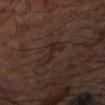The lesion was photographed on a routine skin check and not biopsied; there is no pathology result. The subject is a male aged 63–67. Automated image analysis of the tile measured an eccentricity of roughly 0.95 and a symmetry-axis asymmetry near 0.35. And it measured a border-irregularity rating of about 5/10, a within-lesion color-variation index near 2/10, and peripheral color asymmetry of about 0.5. It also reported a nevus-likeness score of about 0/100 and a detector confidence of about 85 out of 100 that the crop contains a lesion. A close-up tile cropped from a whole-body skin photograph, about 15 mm across. From the arm. Approximately 4.5 mm at its widest. The tile uses cross-polarized illumination.